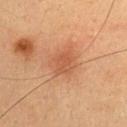Assessment: Captured during whole-body skin photography for melanoma surveillance; the lesion was not biopsied.